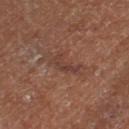subject: female; image source: 15 mm crop, total-body photography; location: the left thigh.